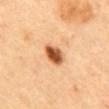Clinical impression: No biopsy was performed on this lesion — it was imaged during a full skin examination and was not determined to be concerning. Image and clinical context: A female subject, approximately 60 years of age. Approximately 3.5 mm at its widest. The lesion is on the mid back. This is a cross-polarized tile. A lesion tile, about 15 mm wide, cut from a 3D total-body photograph.